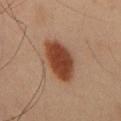Notes:
• workup · imaged on a skin check; not biopsied
• lighting · cross-polarized illumination
• anatomic site · the chest
• diameter · ~6.5 mm (longest diameter)
• patient · male, about 45 years old
• imaging modality · ~15 mm crop, total-body skin-cancer survey